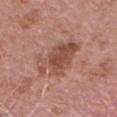Assessment:
No biopsy was performed on this lesion — it was imaged during a full skin examination and was not determined to be concerning.
Context:
The subject is a male aged around 75. The lesion is on the head or neck. This image is a 15 mm lesion crop taken from a total-body photograph.